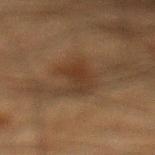<case>
  <biopsy_status>not biopsied; imaged during a skin examination</biopsy_status>
  <lesion_size>
    <long_diameter_mm_approx>4.0</long_diameter_mm_approx>
  </lesion_size>
  <image>
    <source>total-body photography crop</source>
    <field_of_view_mm>15</field_of_view_mm>
  </image>
  <patient>
    <sex>male</sex>
    <age_approx>35</age_approx>
  </patient>
  <site>leg</site>
  <lighting>cross-polarized</lighting>
</case>